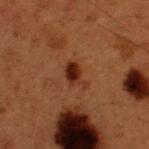Q: Was a biopsy performed?
A: total-body-photography surveillance lesion; no biopsy
Q: What lighting was used for the tile?
A: cross-polarized
Q: Patient demographics?
A: male, about 50 years old
Q: What is the anatomic site?
A: the upper back
Q: What kind of image is this?
A: ~15 mm crop, total-body skin-cancer survey
Q: What is the lesion's diameter?
A: ≈3.5 mm
Q: What did automated image analysis measure?
A: an eccentricity of roughly 0.75 and two-axis asymmetry of about 0.3; a mean CIELAB color near L≈23 a*≈20 b*≈26, roughly 9 lightness units darker than nearby skin, and a lesion-to-skin contrast of about 10 (normalized; higher = more distinct); border irregularity of about 3 on a 0–10 scale